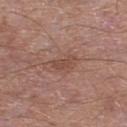A 15 mm close-up tile from a total-body photography series done for melanoma screening.
A male patient in their mid- to late 50s.
From the left lower leg.